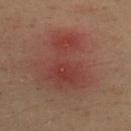Imaged during a routine full-body skin examination; the lesion was not biopsied and no histopathology is available.
A male patient, aged 33 to 37.
This is a cross-polarized tile.
Located on the upper back.
About 7 mm across.
An algorithmic analysis of the crop reported a border-irregularity index near 8/10, internal color variation of about 4 on a 0–10 scale, and peripheral color asymmetry of about 1. The analysis additionally found a classifier nevus-likeness of about 0/100 and a detector confidence of about 100 out of 100 that the crop contains a lesion.
Cropped from a total-body skin-imaging series; the visible field is about 15 mm.